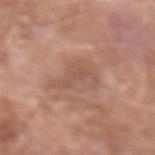notes = imaged on a skin check; not biopsied.